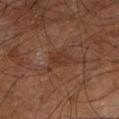biopsy status — no biopsy performed (imaged during a skin exam) | lesion diameter — ~3.5 mm (longest diameter) | body site — the leg | patient — male, aged around 60 | illumination — cross-polarized | acquisition — 15 mm crop, total-body photography.